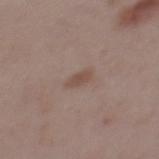No biopsy was performed on this lesion — it was imaged during a full skin examination and was not determined to be concerning. This image is a 15 mm lesion crop taken from a total-body photograph. On the mid back. Captured under white-light illumination. A female patient, about 45 years old. About 2.5 mm across. An algorithmic analysis of the crop reported an outline eccentricity of about 0.85 (0 = round, 1 = elongated) and a shape-asymmetry score of about 0.3 (0 = symmetric). It also reported a lesion color around L≈49 a*≈16 b*≈24 in CIELAB, roughly 7 lightness units darker than nearby skin, and a normalized border contrast of about 6.5. The software also gave a detector confidence of about 100 out of 100 that the crop contains a lesion.